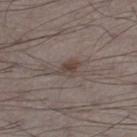{"biopsy_status": "not biopsied; imaged during a skin examination", "lesion_size": {"long_diameter_mm_approx": 3.0}, "lighting": "white-light", "patient": {"sex": "male", "age_approx": 70}, "automated_metrics": {"area_mm2_approx": 4.0, "eccentricity": 0.7, "shape_asymmetry": 0.35, "border_irregularity_0_10": 3.5, "color_variation_0_10": 2.0, "peripheral_color_asymmetry": 0.5, "nevus_likeness_0_100": 35, "lesion_detection_confidence_0_100": 100}, "site": "left lower leg", "image": {"source": "total-body photography crop", "field_of_view_mm": 15}}